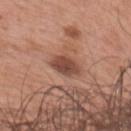workup: catalogued during a skin exam; not biopsied | anatomic site: the right upper arm | patient: male, in their mid- to late 50s | image source: ~15 mm tile from a whole-body skin photo.